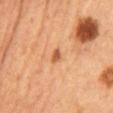From the abdomen. The patient is female. Cropped from a whole-body photographic skin survey; the tile spans about 15 mm.